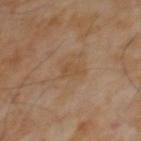A 15 mm crop from a total-body photograph taken for skin-cancer surveillance.
A male patient, roughly 65 years of age.
The tile uses cross-polarized illumination.
The lesion's longest dimension is about 3 mm.